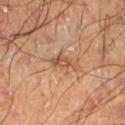| field | value |
|---|---|
| workup | no biopsy performed (imaged during a skin exam) |
| acquisition | ~15 mm crop, total-body skin-cancer survey |
| tile lighting | cross-polarized |
| anatomic site | the left lower leg |
| lesion size | ≈3.5 mm |
| patient | male, aged approximately 60 |